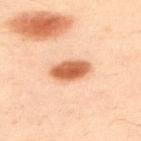Case summary:
• follow-up — imaged on a skin check; not biopsied
• acquisition — 15 mm crop, total-body photography
• image-analysis metrics — a shape eccentricity near 0.85; an average lesion color of about L≈56 a*≈24 b*≈36 (CIELAB), roughly 16 lightness units darker than nearby skin, and a normalized border contrast of about 11
• subject — male, aged 48–52
• lesion diameter — ≈4.5 mm
• tile lighting — cross-polarized illumination
• site — the upper back